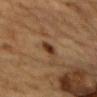- lesion size: ~2.5 mm (longest diameter)
- anatomic site: the abdomen
- image source: 15 mm crop, total-body photography
- subject: male, roughly 85 years of age or older
- tile lighting: cross-polarized
- automated metrics: a peripheral color-asymmetry measure near 0.5